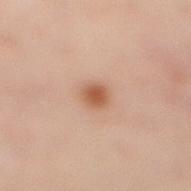A close-up tile cropped from a whole-body skin photograph, about 15 mm across.
The total-body-photography lesion software estimated an area of roughly 4 mm², an outline eccentricity of about 0.35 (0 = round, 1 = elongated), and a shape-asymmetry score of about 0.25 (0 = symmetric). And it measured about 12 CIELAB-L* units darker than the surrounding skin and a lesion-to-skin contrast of about 8.5 (normalized; higher = more distinct). The software also gave an automated nevus-likeness rating near 100 out of 100 and a detector confidence of about 100 out of 100 that the crop contains a lesion.
Approximately 2.5 mm at its widest.
Imaged with cross-polarized lighting.
A female subject, aged 38 to 42.
On the leg.